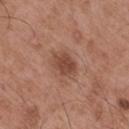* workup: imaged on a skin check; not biopsied
* site: the mid back
* automated metrics: a footprint of about 6.5 mm²; a lesion color around L≈46 a*≈22 b*≈28 in CIELAB, roughly 10 lightness units darker than nearby skin, and a normalized border contrast of about 7.5; a border-irregularity index near 1.5/10, a within-lesion color-variation index near 2.5/10, and a peripheral color-asymmetry measure near 1
* size: ~3.5 mm (longest diameter)
* imaging modality: ~15 mm crop, total-body skin-cancer survey
* patient: male, approximately 55 years of age
* lighting: white-light illumination